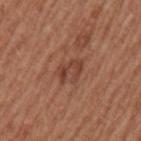• follow-up — no biopsy performed (imaged during a skin exam)
• lighting — white-light illumination
• imaging modality — 15 mm crop, total-body photography
• body site — the left upper arm
• subject — male, aged 53–57
• lesion size — ≈3.5 mm
• automated metrics — a footprint of about 5.5 mm² and an outline eccentricity of about 0.75 (0 = round, 1 = elongated); a border-irregularity rating of about 5.5/10 and a color-variation rating of about 3/10